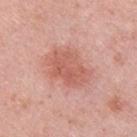| feature | finding |
|---|---|
| follow-up | catalogued during a skin exam; not biopsied |
| patient | male, roughly 25 years of age |
| body site | the upper back |
| image | total-body-photography crop, ~15 mm field of view |
| lesion diameter | ≈5.5 mm |